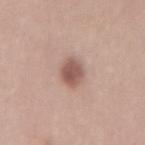Findings:
• biopsy status: imaged on a skin check; not biopsied
• automated metrics: an area of roughly 6 mm², a shape eccentricity near 0.8, and a shape-asymmetry score of about 0.25 (0 = symmetric); a lesion color around L≈54 a*≈20 b*≈24 in CIELAB, about 13 CIELAB-L* units darker than the surrounding skin, and a normalized border contrast of about 9
• anatomic site: the back
• image source: 15 mm crop, total-body photography
• subject: female, approximately 25 years of age